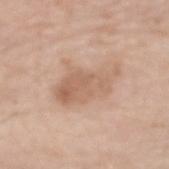Assessment:
No biopsy was performed on this lesion — it was imaged during a full skin examination and was not determined to be concerning.
Acquisition and patient details:
From the right forearm. A male patient approximately 80 years of age. Measured at roughly 6.5 mm in maximum diameter. The lesion-visualizer software estimated a footprint of about 16 mm², a shape eccentricity near 0.85, and a shape-asymmetry score of about 0.35 (0 = symmetric). And it measured a border-irregularity index near 5/10, a color-variation rating of about 4.5/10, and radial color variation of about 1.5. A 15 mm close-up extracted from a 3D total-body photography capture. This is a white-light tile.